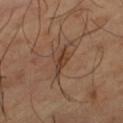Case summary:
– diameter · ≈4 mm
– image source · ~15 mm crop, total-body skin-cancer survey
– illumination · cross-polarized
– location · the left thigh
– subject · male, aged 63 to 67